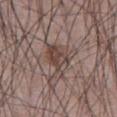follow-up: imaged on a skin check; not biopsied
image source: ~15 mm tile from a whole-body skin photo
lesion diameter: ≈4.5 mm
site: the abdomen
lighting: white-light illumination
subject: male, roughly 65 years of age